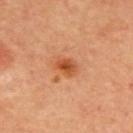Case summary:
- workup · imaged on a skin check; not biopsied
- acquisition · total-body-photography crop, ~15 mm field of view
- site · the upper back
- size · about 2.5 mm
- illumination · cross-polarized illumination
- patient · male, aged approximately 65
- automated metrics · a lesion area of about 4.5 mm²; a lesion color around L≈53 a*≈30 b*≈41 in CIELAB, about 11 CIELAB-L* units darker than the surrounding skin, and a lesion-to-skin contrast of about 8 (normalized; higher = more distinct); radial color variation of about 1